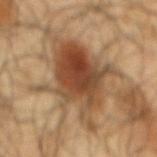workup = no biopsy performed (imaged during a skin exam)
imaging modality = ~15 mm crop, total-body skin-cancer survey
body site = the mid back
subject = male, in their mid- to late 60s